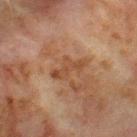Part of a total-body skin-imaging series; this lesion was reviewed on a skin check and was not flagged for biopsy. A 15 mm close-up tile from a total-body photography series done for melanoma screening. From the front of the torso. A male subject approximately 65 years of age.